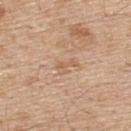{"biopsy_status": "not biopsied; imaged during a skin examination", "lesion_size": {"long_diameter_mm_approx": 3.0}, "lighting": "white-light", "automated_metrics": {"area_mm2_approx": 3.0, "eccentricity": 0.85, "shape_asymmetry": 0.5, "nevus_likeness_0_100": 0, "lesion_detection_confidence_0_100": 100}, "patient": {"sex": "male", "age_approx": 70}, "image": {"source": "total-body photography crop", "field_of_view_mm": 15}, "site": "upper back"}